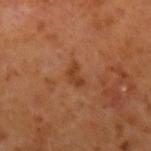Part of a total-body skin-imaging series; this lesion was reviewed on a skin check and was not flagged for biopsy. A 15 mm close-up extracted from a 3D total-body photography capture. The patient is a male roughly 60 years of age. The lesion is located on the left forearm. Captured under cross-polarized illumination. The lesion's longest dimension is about 2.5 mm. An algorithmic analysis of the crop reported an area of roughly 3 mm² and a shape-asymmetry score of about 0.45 (0 = symmetric). The analysis additionally found a lesion color around L≈37 a*≈23 b*≈33 in CIELAB and roughly 8 lightness units darker than nearby skin. The software also gave a border-irregularity rating of about 4/10, a within-lesion color-variation index near 0.5/10, and peripheral color asymmetry of about 0. The software also gave a classifier nevus-likeness of about 0/100 and lesion-presence confidence of about 100/100.